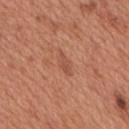Impression: The lesion was tiled from a total-body skin photograph and was not biopsied. Context: A 15 mm close-up extracted from a 3D total-body photography capture. Located on the upper back. A male subject, aged approximately 65.